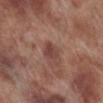Imaged during a routine full-body skin examination; the lesion was not biopsied and no histopathology is available.